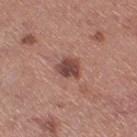Notes:
- workup · total-body-photography surveillance lesion; no biopsy
- tile lighting · white-light
- site · the leg
- image-analysis metrics · a lesion color around L≈44 a*≈23 b*≈24 in CIELAB, a lesion–skin lightness drop of about 14, and a normalized border contrast of about 10; an automated nevus-likeness rating near 65 out of 100 and a lesion-detection confidence of about 100/100
- image source · total-body-photography crop, ~15 mm field of view
- lesion size · ≈2.5 mm
- patient · female, about 30 years old